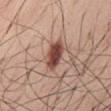| field | value |
|---|---|
| location | the abdomen |
| patient | male, aged 53 to 57 |
| lesion size | ≈3.5 mm |
| imaging modality | 15 mm crop, total-body photography |
| lighting | white-light illumination |